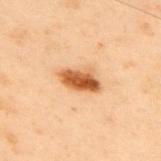Part of a total-body skin-imaging series; this lesion was reviewed on a skin check and was not flagged for biopsy. The lesion's longest dimension is about 4 mm. A 15 mm close-up tile from a total-body photography series done for melanoma screening. Imaged with cross-polarized lighting. Automated tile analysis of the lesion measured a lesion-detection confidence of about 100/100. On the upper back. A male subject aged around 55.